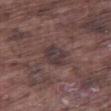Clinical impression:
Captured during whole-body skin photography for melanoma surveillance; the lesion was not biopsied.
Acquisition and patient details:
The patient is a male aged around 75. The recorded lesion diameter is about 3.5 mm. A region of skin cropped from a whole-body photographic capture, roughly 15 mm wide. The lesion-visualizer software estimated a border-irregularity index near 2.5/10, internal color variation of about 2.5 on a 0–10 scale, and peripheral color asymmetry of about 1. The analysis additionally found an automated nevus-likeness rating near 0 out of 100. This is a white-light tile. From the leg.